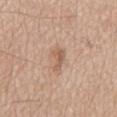Captured during whole-body skin photography for melanoma surveillance; the lesion was not biopsied.
Captured under white-light illumination.
The subject is a male approximately 75 years of age.
On the mid back.
A 15 mm crop from a total-body photograph taken for skin-cancer surveillance.